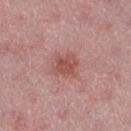Recorded during total-body skin imaging; not selected for excision or biopsy. A female patient aged 43–47. The recorded lesion diameter is about 3 mm. A lesion tile, about 15 mm wide, cut from a 3D total-body photograph. The lesion is on the right thigh.